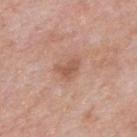| key | value |
|---|---|
| body site | the mid back |
| tile lighting | white-light illumination |
| image source | ~15 mm tile from a whole-body skin photo |
| lesion size | ~3 mm (longest diameter) |
| subject | male, aged 53 to 57 |